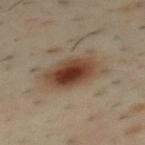The lesion was tiled from a total-body skin photograph and was not biopsied. About 6 mm across. A roughly 15 mm field-of-view crop from a total-body skin photograph. Captured under cross-polarized illumination. The lesion is located on the mid back. A male patient, aged around 40. The total-body-photography lesion software estimated an area of roughly 17 mm², a shape eccentricity near 0.8, and a shape-asymmetry score of about 0.15 (0 = symmetric). And it measured border irregularity of about 2.5 on a 0–10 scale, a color-variation rating of about 7.5/10, and radial color variation of about 2. The software also gave a classifier nevus-likeness of about 100/100 and a lesion-detection confidence of about 100/100.